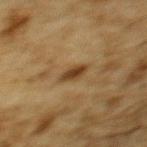notes — imaged on a skin check; not biopsied | body site — the upper back | imaging modality — ~15 mm tile from a whole-body skin photo | subject — male, roughly 85 years of age or older.